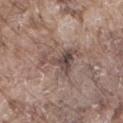Q: Is there a histopathology result?
A: catalogued during a skin exam; not biopsied
Q: How was the tile lit?
A: white-light illumination
Q: Lesion size?
A: about 5 mm
Q: What kind of image is this?
A: ~15 mm tile from a whole-body skin photo
Q: What did automated image analysis measure?
A: a footprint of about 11 mm², an outline eccentricity of about 0.75 (0 = round, 1 = elongated), and a shape-asymmetry score of about 0.7 (0 = symmetric); a lesion color around L≈48 a*≈15 b*≈20 in CIELAB, a lesion–skin lightness drop of about 10, and a normalized border contrast of about 8; a border-irregularity index near 8.5/10, a color-variation rating of about 6.5/10, and peripheral color asymmetry of about 2; a classifier nevus-likeness of about 0/100 and a lesion-detection confidence of about 80/100
Q: Lesion location?
A: the right thigh
Q: What are the patient's age and sex?
A: male, about 75 years old